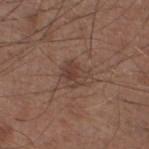<tbp_lesion>
<biopsy_status>not biopsied; imaged during a skin examination</biopsy_status>
<image>
  <source>total-body photography crop</source>
  <field_of_view_mm>15</field_of_view_mm>
</image>
<lighting>white-light</lighting>
<automated_metrics>
  <area_mm2_approx>5.5</area_mm2_approx>
  <eccentricity>0.65</eccentricity>
  <cielab_L>40</cielab_L>
  <cielab_a>18</cielab_a>
  <cielab_b>24</cielab_b>
  <nevus_likeness_0_100>60</nevus_likeness_0_100>
  <lesion_detection_confidence_0_100>100</lesion_detection_confidence_0_100>
</automated_metrics>
<lesion_size>
  <long_diameter_mm_approx>3.0</long_diameter_mm_approx>
</lesion_size>
<site>leg</site>
<patient>
  <sex>male</sex>
  <age_approx>60</age_approx>
</patient>
</tbp_lesion>